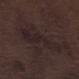– follow-up · catalogued during a skin exam; not biopsied
– location · the leg
– size · about 7 mm
– subject · male, aged 68–72
– image source · 15 mm crop, total-body photography